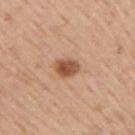A male subject, about 75 years old. A close-up tile cropped from a whole-body skin photograph, about 15 mm across. Measured at roughly 3 mm in maximum diameter. The lesion-visualizer software estimated a footprint of about 5 mm², an outline eccentricity of about 0.7 (0 = round, 1 = elongated), and a symmetry-axis asymmetry near 0.2. And it measured a lesion–skin lightness drop of about 14 and a normalized lesion–skin contrast near 9.5. The software also gave a border-irregularity rating of about 2/10, a within-lesion color-variation index near 3.5/10, and a peripheral color-asymmetry measure near 1. The software also gave a nevus-likeness score of about 100/100 and lesion-presence confidence of about 100/100. From the right upper arm.